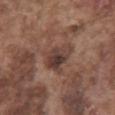No biopsy was performed on this lesion — it was imaged during a full skin examination and was not determined to be concerning.
The patient is a male aged around 75.
On the abdomen.
The lesion-visualizer software estimated a lesion area of about 10 mm², a shape eccentricity near 0.65, and a shape-asymmetry score of about 0.35 (0 = symmetric). The software also gave about 10 CIELAB-L* units darker than the surrounding skin and a lesion-to-skin contrast of about 8.5 (normalized; higher = more distinct). The analysis additionally found a classifier nevus-likeness of about 15/100 and a lesion-detection confidence of about 100/100.
Approximately 4 mm at its widest.
Imaged with white-light lighting.
A 15 mm close-up extracted from a 3D total-body photography capture.